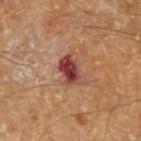Clinical impression:
Part of a total-body skin-imaging series; this lesion was reviewed on a skin check and was not flagged for biopsy.
Background:
The tile uses cross-polarized illumination. An algorithmic analysis of the crop reported a footprint of about 6.5 mm² and an outline eccentricity of about 0.8 (0 = round, 1 = elongated). The software also gave a classifier nevus-likeness of about 0/100 and a detector confidence of about 100 out of 100 that the crop contains a lesion. A male subject, aged 68 to 72. Approximately 3.5 mm at its widest. Located on the arm. A lesion tile, about 15 mm wide, cut from a 3D total-body photograph.